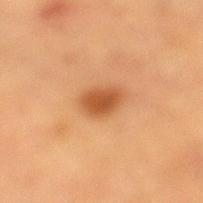follow-up=catalogued during a skin exam; not biopsied | size=~3.5 mm (longest diameter) | site=the left lower leg | acquisition=15 mm crop, total-body photography | TBP lesion metrics=an area of roughly 6.5 mm² and a shape eccentricity near 0.7; a border-irregularity rating of about 2.5/10, a color-variation rating of about 2/10, and peripheral color asymmetry of about 0.5; a nevus-likeness score of about 95/100 | subject=male, roughly 85 years of age or older.